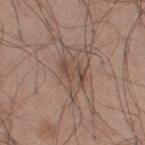| feature | finding |
|---|---|
| follow-up | imaged on a skin check; not biopsied |
| automated lesion analysis | a footprint of about 9.5 mm²; an average lesion color of about L≈49 a*≈16 b*≈24 (CIELAB), about 8 CIELAB-L* units darker than the surrounding skin, and a lesion-to-skin contrast of about 6 (normalized; higher = more distinct); a color-variation rating of about 4.5/10 and peripheral color asymmetry of about 1.5 |
| illumination | white-light illumination |
| body site | the right thigh |
| imaging modality | total-body-photography crop, ~15 mm field of view |
| lesion size | ~5.5 mm (longest diameter) |
| patient | male, about 45 years old |